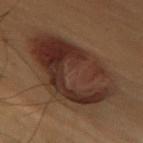{"biopsy_status": "not biopsied; imaged during a skin examination", "site": "chest", "patient": {"sex": "female", "age_approx": 60}, "image": {"source": "total-body photography crop", "field_of_view_mm": 15}}